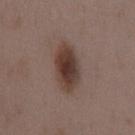The lesion was photographed on a routine skin check and not biopsied; there is no pathology result.
A female subject, in their mid- to late 30s.
The lesion is located on the mid back.
A 15 mm close-up tile from a total-body photography series done for melanoma screening.
Captured under white-light illumination.
The total-body-photography lesion software estimated a footprint of about 14 mm², an outline eccentricity of about 0.9 (0 = round, 1 = elongated), and a symmetry-axis asymmetry near 0.15. It also reported an average lesion color of about L≈38 a*≈16 b*≈22 (CIELAB), about 12 CIELAB-L* units darker than the surrounding skin, and a normalized lesion–skin contrast near 10.5. It also reported border irregularity of about 2 on a 0–10 scale, a color-variation rating of about 5.5/10, and radial color variation of about 1.5. And it measured a classifier nevus-likeness of about 100/100 and a detector confidence of about 100 out of 100 that the crop contains a lesion.